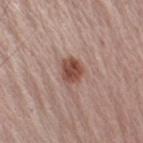- workup — imaged on a skin check; not biopsied
- tile lighting — white-light illumination
- lesion diameter — ≈3 mm
- location — the right upper arm
- imaging modality — ~15 mm crop, total-body skin-cancer survey
- patient — male, approximately 65 years of age